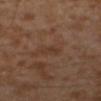Clinical impression:
No biopsy was performed on this lesion — it was imaged during a full skin examination and was not determined to be concerning.
Acquisition and patient details:
A male subject, aged 28–32. The tile uses cross-polarized illumination. About 2.5 mm across. The lesion is located on the left lower leg. Cropped from a total-body skin-imaging series; the visible field is about 15 mm.